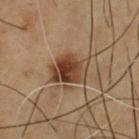Impression:
The lesion was photographed on a routine skin check and not biopsied; there is no pathology result.
Acquisition and patient details:
A male patient, in their mid- to late 50s. The lesion is on the front of the torso. A close-up tile cropped from a whole-body skin photograph, about 15 mm across. Longest diameter approximately 4 mm. An algorithmic analysis of the crop reported an area of roughly 11 mm², an outline eccentricity of about 0.7 (0 = round, 1 = elongated), and a shape-asymmetry score of about 0.3 (0 = symmetric). And it measured a lesion–skin lightness drop of about 11 and a normalized lesion–skin contrast near 10.5. The software also gave a color-variation rating of about 7/10 and radial color variation of about 2.5. The software also gave a lesion-detection confidence of about 100/100.